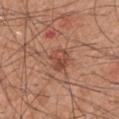{
  "image": {
    "source": "total-body photography crop",
    "field_of_view_mm": 15
  },
  "site": "right upper arm",
  "patient": {
    "sex": "male",
    "age_approx": 60
  },
  "automated_metrics": {
    "vs_skin_darker_L": 9.0,
    "vs_skin_contrast_norm": 6.5,
    "border_irregularity_0_10": 1.5,
    "color_variation_0_10": 5.0,
    "peripheral_color_asymmetry": 2.0
  }
}